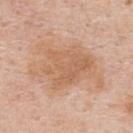Assessment: Part of a total-body skin-imaging series; this lesion was reviewed on a skin check and was not flagged for biopsy. Acquisition and patient details: Automated tile analysis of the lesion measured a border-irregularity rating of about 5.5/10, a within-lesion color-variation index near 3.5/10, and peripheral color asymmetry of about 1. Approximately 8.5 mm at its widest. A male subject aged approximately 60. The lesion is on the upper back. This is a white-light tile. A 15 mm crop from a total-body photograph taken for skin-cancer surveillance.